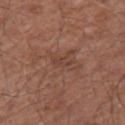Assessment: Recorded during total-body skin imaging; not selected for excision or biopsy. Background: Measured at roughly 2.5 mm in maximum diameter. A male subject aged 53 to 57. On the right forearm. This image is a 15 mm lesion crop taken from a total-body photograph. This is a white-light tile. Automated tile analysis of the lesion measured an outline eccentricity of about 0.9 (0 = round, 1 = elongated) and a symmetry-axis asymmetry near 0.3. And it measured a mean CIELAB color near L≈42 a*≈21 b*≈26 and roughly 6 lightness units darker than nearby skin.